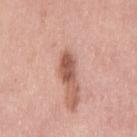Impression: Recorded during total-body skin imaging; not selected for excision or biopsy. Acquisition and patient details: Imaged with white-light lighting. About 3.5 mm across. From the leg. A female patient aged 48–52. This image is a 15 mm lesion crop taken from a total-body photograph.